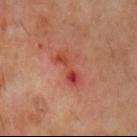{
  "biopsy_status": "not biopsied; imaged during a skin examination",
  "automated_metrics": {
    "area_mm2_approx": 5.5,
    "eccentricity": 0.95,
    "shape_asymmetry": 0.3,
    "border_irregularity_0_10": 4.5,
    "color_variation_0_10": 9.0,
    "lesion_detection_confidence_0_100": 100
  },
  "lesion_size": {
    "long_diameter_mm_approx": 4.5
  },
  "site": "left upper arm",
  "image": {
    "source": "total-body photography crop",
    "field_of_view_mm": 15
  },
  "lighting": "cross-polarized",
  "patient": {
    "sex": "male",
    "age_approx": 60
  }
}